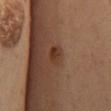follow-up=imaged on a skin check; not biopsied | subject=female, aged around 55 | image=15 mm crop, total-body photography | location=the mid back | lighting=cross-polarized.